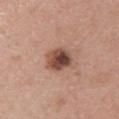No biopsy was performed on this lesion — it was imaged during a full skin examination and was not determined to be concerning. A female patient, aged approximately 40. From the right upper arm. A region of skin cropped from a whole-body photographic capture, roughly 15 mm wide. The lesion's longest dimension is about 3.5 mm. Imaged with white-light lighting.